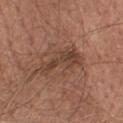workup = total-body-photography surveillance lesion; no biopsy | acquisition = ~15 mm tile from a whole-body skin photo | image-analysis metrics = a lesion color around L≈42 a*≈19 b*≈27 in CIELAB and a lesion–skin lightness drop of about 9; a classifier nevus-likeness of about 20/100 and a lesion-detection confidence of about 85/100 | site = the head or neck | illumination = white-light | subject = female, aged around 65 | size = ~7 mm (longest diameter).